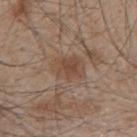Background:
A 15 mm crop from a total-body photograph taken for skin-cancer surveillance. The lesion-visualizer software estimated a footprint of about 10 mm², an eccentricity of roughly 0.65, and two-axis asymmetry of about 0.2. The patient is a male aged 43 to 47. Approximately 4 mm at its widest. The lesion is on the mid back. This is a white-light tile.